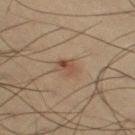Impression: Part of a total-body skin-imaging series; this lesion was reviewed on a skin check and was not flagged for biopsy. Image and clinical context: Captured under cross-polarized illumination. The recorded lesion diameter is about 2.5 mm. A male subject, aged around 35. A lesion tile, about 15 mm wide, cut from a 3D total-body photograph. On the right thigh.